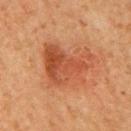Q: Was this lesion biopsied?
A: total-body-photography surveillance lesion; no biopsy
Q: Illumination type?
A: cross-polarized illumination
Q: What kind of image is this?
A: ~15 mm tile from a whole-body skin photo
Q: Lesion location?
A: the mid back
Q: Patient demographics?
A: male, in their mid- to late 60s
Q: Lesion size?
A: ≈6 mm
Q: Automated lesion metrics?
A: a lesion color around L≈44 a*≈26 b*≈34 in CIELAB, about 9 CIELAB-L* units darker than the surrounding skin, and a normalized border contrast of about 7; a border-irregularity rating of about 6/10, a color-variation rating of about 5.5/10, and peripheral color asymmetry of about 2; a classifier nevus-likeness of about 10/100 and a lesion-detection confidence of about 100/100